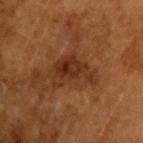workup = imaged on a skin check; not biopsied
automated metrics = a within-lesion color-variation index near 5/10 and radial color variation of about 1.5; a nevus-likeness score of about 25/100 and lesion-presence confidence of about 100/100
subject = male, aged approximately 65
tile lighting = cross-polarized
imaging modality = ~15 mm tile from a whole-body skin photo
lesion size = ≈5.5 mm